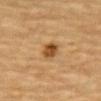Q: What is the lesion's diameter?
A: ≈2.5 mm
Q: How was this image acquired?
A: 15 mm crop, total-body photography
Q: What is the anatomic site?
A: the front of the torso
Q: How was the tile lit?
A: cross-polarized
Q: What are the patient's age and sex?
A: male, about 85 years old or older